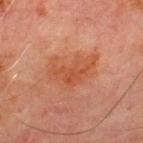Part of a total-body skin-imaging series; this lesion was reviewed on a skin check and was not flagged for biopsy. A male subject roughly 70 years of age. A region of skin cropped from a whole-body photographic capture, roughly 15 mm wide. The lesion's longest dimension is about 5.5 mm. An algorithmic analysis of the crop reported border irregularity of about 5.5 on a 0–10 scale and peripheral color asymmetry of about 1. On the front of the torso. Imaged with cross-polarized lighting.